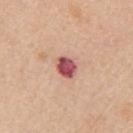Q: Is there a histopathology result?
A: no biopsy performed (imaged during a skin exam)
Q: How was this image acquired?
A: total-body-photography crop, ~15 mm field of view
Q: What is the anatomic site?
A: the right upper arm
Q: Patient demographics?
A: male, aged 63 to 67
Q: How large is the lesion?
A: ~3 mm (longest diameter)
Q: What did automated image analysis measure?
A: a footprint of about 5.5 mm², an outline eccentricity of about 0.55 (0 = round, 1 = elongated), and a symmetry-axis asymmetry near 0.15; border irregularity of about 1.5 on a 0–10 scale, a within-lesion color-variation index near 7/10, and peripheral color asymmetry of about 2.5
Q: Illumination type?
A: white-light illumination